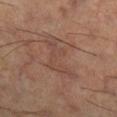Part of a total-body skin-imaging series; this lesion was reviewed on a skin check and was not flagged for biopsy.